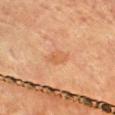workup = total-body-photography surveillance lesion; no biopsy | site = the chest | patient = female, aged 78–82 | lighting = cross-polarized | TBP lesion metrics = a mean CIELAB color near L≈56 a*≈23 b*≈37, a lesion–skin lightness drop of about 6, and a normalized lesion–skin contrast near 5 | acquisition = total-body-photography crop, ~15 mm field of view.